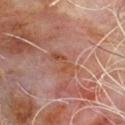biopsy_status: not biopsied; imaged during a skin examination
lighting: cross-polarized
lesion_size:
  long_diameter_mm_approx: 4.0
site: chest
patient:
  sex: male
  age_approx: 65
image:
  source: total-body photography crop
  field_of_view_mm: 15
automated_metrics:
  area_mm2_approx: 5.0
  eccentricity: 0.9
  nevus_likeness_0_100: 0